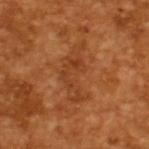Assessment: The lesion was photographed on a routine skin check and not biopsied; there is no pathology result. Background: The subject is a male in their mid-60s. A 15 mm close-up extracted from a 3D total-body photography capture.